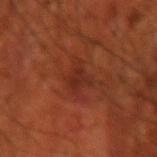Impression:
Captured during whole-body skin photography for melanoma surveillance; the lesion was not biopsied.
Background:
The recorded lesion diameter is about 3.5 mm. On the right forearm. This is a cross-polarized tile. A male patient, aged 68–72. A lesion tile, about 15 mm wide, cut from a 3D total-body photograph.